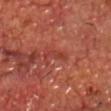Imaged during a routine full-body skin examination; the lesion was not biopsied and no histopathology is available.
From the chest.
A 15 mm close-up extracted from a 3D total-body photography capture.
The total-body-photography lesion software estimated lesion-presence confidence of about 90/100.
Captured under cross-polarized illumination.
The subject is a male aged 68–72.